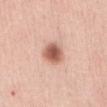Case summary:
– biopsy status: no biopsy performed (imaged during a skin exam)
– lesion size: ~3 mm (longest diameter)
– image-analysis metrics: a footprint of about 7 mm², a shape eccentricity near 0.25, and two-axis asymmetry of about 0.15; an average lesion color of about L≈60 a*≈24 b*≈29 (CIELAB), roughly 16 lightness units darker than nearby skin, and a lesion-to-skin contrast of about 10 (normalized; higher = more distinct); border irregularity of about 1.5 on a 0–10 scale and peripheral color asymmetry of about 1; an automated nevus-likeness rating near 100 out of 100 and a detector confidence of about 100 out of 100 that the crop contains a lesion
– lighting: white-light illumination
– acquisition: 15 mm crop, total-body photography
– subject: female, aged around 45
– location: the abdomen